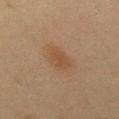  biopsy_status: not biopsied; imaged during a skin examination
  site: upper back
  patient:
    sex: male
    age_approx: 45
  lighting: cross-polarized
  image:
    source: total-body photography crop
    field_of_view_mm: 15